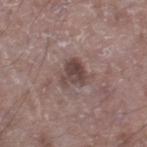<record>
<biopsy_status>not biopsied; imaged during a skin examination</biopsy_status>
<lesion_size>
  <long_diameter_mm_approx>3.0</long_diameter_mm_approx>
</lesion_size>
<patient>
  <sex>male</sex>
  <age_approx>50</age_approx>
</patient>
<image>
  <source>total-body photography crop</source>
  <field_of_view_mm>15</field_of_view_mm>
</image>
<automated_metrics>
  <area_mm2_approx>6.0</area_mm2_approx>
  <shape_asymmetry>0.45</shape_asymmetry>
  <cielab_L>43</cielab_L>
  <cielab_a>16</cielab_a>
  <cielab_b>18</cielab_b>
  <vs_skin_contrast_norm>8.5</vs_skin_contrast_norm>
  <nevus_likeness_0_100>0</nevus_likeness_0_100>
  <lesion_detection_confidence_0_100>100</lesion_detection_confidence_0_100>
</automated_metrics>
<site>right lower leg</site>
</record>